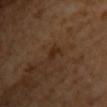notes=imaged on a skin check; not biopsied | diameter=≈2.5 mm | subject=female | anatomic site=the upper back | image-analysis metrics=a footprint of about 2.5 mm², a shape eccentricity near 0.85, and a symmetry-axis asymmetry near 0.45; a mean CIELAB color near L≈27 a*≈18 b*≈28, about 6 CIELAB-L* units darker than the surrounding skin, and a normalized border contrast of about 7; an automated nevus-likeness rating near 20 out of 100 and a lesion-detection confidence of about 100/100 | imaging modality=~15 mm tile from a whole-body skin photo.